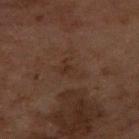The lesion was tiled from a total-body skin photograph and was not biopsied.
A lesion tile, about 15 mm wide, cut from a 3D total-body photograph.
The recorded lesion diameter is about 3.5 mm.
Automated image analysis of the tile measured a lesion–skin lightness drop of about 4 and a lesion-to-skin contrast of about 5 (normalized; higher = more distinct).
Captured under cross-polarized illumination.
On the arm.
The subject is a female roughly 60 years of age.